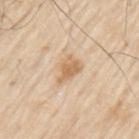notes: no biopsy performed (imaged during a skin exam); size: ~3.5 mm (longest diameter); subject: male, about 80 years old; image source: ~15 mm crop, total-body skin-cancer survey; anatomic site: the left upper arm; lighting: white-light illumination.